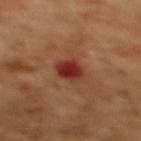Assessment: Part of a total-body skin-imaging series; this lesion was reviewed on a skin check and was not flagged for biopsy. Clinical summary: Captured under cross-polarized illumination. The lesion's longest dimension is about 3.5 mm. A 15 mm close-up tile from a total-body photography series done for melanoma screening. On the upper back. A female patient approximately 55 years of age.